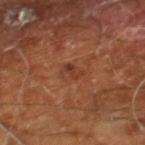Q: Was this lesion biopsied?
A: catalogued during a skin exam; not biopsied
Q: What are the patient's age and sex?
A: male, in their 60s
Q: What kind of image is this?
A: ~15 mm crop, total-body skin-cancer survey
Q: Lesion size?
A: about 2.5 mm
Q: Where on the body is the lesion?
A: the right leg